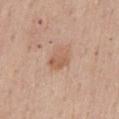Recorded during total-body skin imaging; not selected for excision or biopsy. A female subject, in their mid-60s. A lesion tile, about 15 mm wide, cut from a 3D total-body photograph. Located on the back. This is a white-light tile. Approximately 3 mm at its widest. An algorithmic analysis of the crop reported a normalized border contrast of about 6. The analysis additionally found a classifier nevus-likeness of about 15/100.